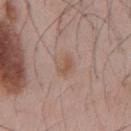Clinical impression:
The lesion was tiled from a total-body skin photograph and was not biopsied.
Acquisition and patient details:
The lesion's longest dimension is about 2.5 mm. The lesion is on the chest. The patient is a male about 55 years old. A region of skin cropped from a whole-body photographic capture, roughly 15 mm wide. The tile uses white-light illumination.